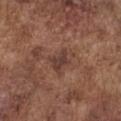Clinical impression: This lesion was catalogued during total-body skin photography and was not selected for biopsy. Context: A 15 mm close-up tile from a total-body photography series done for melanoma screening. A male patient about 75 years old. The lesion is on the chest.